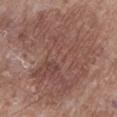follow-up: no biopsy performed (imaged during a skin exam) | tile lighting: white-light illumination | location: the left lower leg | lesion size: ~11.5 mm (longest diameter) | image source: ~15 mm crop, total-body skin-cancer survey | subject: male, aged approximately 85 or older | TBP lesion metrics: an area of roughly 60 mm², a shape eccentricity near 0.65, and a symmetry-axis asymmetry near 0.5; a border-irregularity index near 9/10, a within-lesion color-variation index near 5/10, and radial color variation of about 2.